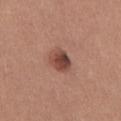{
  "biopsy_status": "not biopsied; imaged during a skin examination",
  "lighting": "white-light",
  "patient": {
    "sex": "female",
    "age_approx": 25
  },
  "site": "abdomen",
  "image": {
    "source": "total-body photography crop",
    "field_of_view_mm": 15
  },
  "lesion_size": {
    "long_diameter_mm_approx": 3.0
  },
  "automated_metrics": {
    "vs_skin_darker_L": 13.0,
    "border_irregularity_0_10": 1.0,
    "color_variation_0_10": 5.5,
    "peripheral_color_asymmetry": 2.0,
    "nevus_likeness_0_100": 90,
    "lesion_detection_confidence_0_100": 100
  }
}